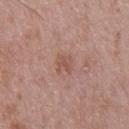Imaged during a routine full-body skin examination; the lesion was not biopsied and no histopathology is available.
The lesion is on the right thigh.
Cropped from a whole-body photographic skin survey; the tile spans about 15 mm.
A male subject, aged approximately 50.